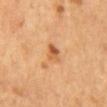The lesion was tiled from a total-body skin photograph and was not biopsied. This image is a 15 mm lesion crop taken from a total-body photograph. A male subject aged around 65. The recorded lesion diameter is about 3 mm. The lesion is on the mid back.